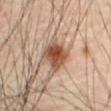Impression: Recorded during total-body skin imaging; not selected for excision or biopsy. Acquisition and patient details: Cropped from a whole-body photographic skin survey; the tile spans about 15 mm. The subject is a male approximately 55 years of age. Automated image analysis of the tile measured an area of roughly 9.5 mm² and a shape-asymmetry score of about 0.2 (0 = symmetric). The software also gave a lesion–skin lightness drop of about 16 and a lesion-to-skin contrast of about 10.5 (normalized; higher = more distinct). The lesion is on the front of the torso. Imaged with cross-polarized lighting.